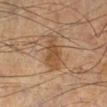Case summary:
- size — ≈4.5 mm
- automated metrics — internal color variation of about 3 on a 0–10 scale and peripheral color asymmetry of about 1
- patient — male, roughly 55 years of age
- imaging modality — total-body-photography crop, ~15 mm field of view
- body site — the right lower leg